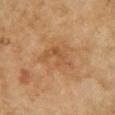Context:
Measured at roughly 4.5 mm in maximum diameter. The patient is a female aged approximately 60. The lesion is located on the chest. A lesion tile, about 15 mm wide, cut from a 3D total-body photograph. Imaged with cross-polarized lighting.